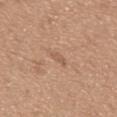workup = no biopsy performed (imaged during a skin exam) | tile lighting = white-light | automated lesion analysis = a mean CIELAB color near L≈57 a*≈20 b*≈31, about 7 CIELAB-L* units darker than the surrounding skin, and a normalized lesion–skin contrast near 5; a color-variation rating of about 0/10 | size = about 2.5 mm | acquisition = ~15 mm crop, total-body skin-cancer survey | site = the mid back | patient = male, aged 63 to 67.